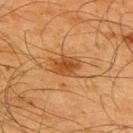Q: Is there a histopathology result?
A: no biopsy performed (imaged during a skin exam)